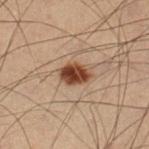| feature | finding |
|---|---|
| image-analysis metrics | an outline eccentricity of about 0.7 (0 = round, 1 = elongated) and two-axis asymmetry of about 0.15; a border-irregularity index near 1.5/10, internal color variation of about 4 on a 0–10 scale, and a peripheral color-asymmetry measure near 1.5; a classifier nevus-likeness of about 100/100 |
| body site | the left lower leg |
| patient | male, aged approximately 55 |
| tile lighting | cross-polarized |
| diameter | ~3.5 mm (longest diameter) |
| image source | 15 mm crop, total-body photography |